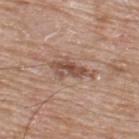Impression:
Captured during whole-body skin photography for melanoma surveillance; the lesion was not biopsied.
Image and clinical context:
Longest diameter approximately 5 mm. Located on the back. This image is a 15 mm lesion crop taken from a total-body photograph. A male patient, in their mid-60s.